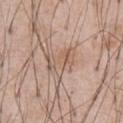| field | value |
|---|---|
| notes | no biopsy performed (imaged during a skin exam) |
| size | ≈4 mm |
| imaging modality | 15 mm crop, total-body photography |
| lighting | white-light |
| automated metrics | an average lesion color of about L≈58 a*≈17 b*≈27 (CIELAB), a lesion–skin lightness drop of about 7, and a normalized lesion–skin contrast near 5; lesion-presence confidence of about 95/100 |
| subject | male, aged 53–57 |
| location | the front of the torso |